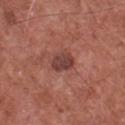Part of a total-body skin-imaging series; this lesion was reviewed on a skin check and was not flagged for biopsy. The tile uses white-light illumination. An algorithmic analysis of the crop reported an average lesion color of about L≈41 a*≈24 b*≈23 (CIELAB), roughly 11 lightness units darker than nearby skin, and a normalized lesion–skin contrast near 8.5. The analysis additionally found border irregularity of about 1.5 on a 0–10 scale and a peripheral color-asymmetry measure near 0.5. A male subject aged 73 to 77. This image is a 15 mm lesion crop taken from a total-body photograph. From the front of the torso. About 3 mm across.